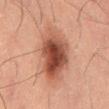| field | value |
|---|---|
| workup | catalogued during a skin exam; not biopsied |
| tile lighting | cross-polarized illumination |
| automated lesion analysis | an area of roughly 22 mm², an eccentricity of roughly 0.9, and a symmetry-axis asymmetry near 0.2; a border-irregularity index near 3/10, a within-lesion color-variation index near 8/10, and radial color variation of about 2.5 |
| site | the front of the torso |
| subject | male, roughly 55 years of age |
| size | ~8 mm (longest diameter) |
| imaging modality | ~15 mm tile from a whole-body skin photo |